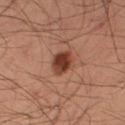Findings:
- body site: the right thigh
- subject: male, about 35 years old
- diameter: about 3 mm
- illumination: cross-polarized illumination
- image: total-body-photography crop, ~15 mm field of view